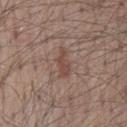The lesion was photographed on a routine skin check and not biopsied; there is no pathology result. A male subject, about 65 years old. A 15 mm close-up extracted from a 3D total-body photography capture. On the back.